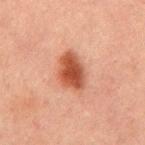  biopsy_status: not biopsied; imaged during a skin examination
  image:
    source: total-body photography crop
    field_of_view_mm: 15
  site: back
  lighting: cross-polarized
  patient:
    sex: male
    age_approx: 50
  automated_metrics:
    area_mm2_approx: 10.0
    eccentricity: 0.7
    shape_asymmetry: 0.2
    cielab_L: 41
    cielab_a: 24
    cielab_b: 29
    vs_skin_darker_L: 12.0
    vs_skin_contrast_norm: 10.0
    border_irregularity_0_10: 2.0
    color_variation_0_10: 4.5
    peripheral_color_asymmetry: 1.0
    nevus_likeness_0_100: 100